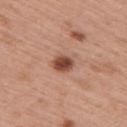| field | value |
|---|---|
| notes | catalogued during a skin exam; not biopsied |
| anatomic site | the upper back |
| lighting | white-light |
| image-analysis metrics | a lesion area of about 4.5 mm² and a shape-asymmetry score of about 0.2 (0 = symmetric); a nevus-likeness score of about 100/100 |
| subject | male, aged approximately 60 |
| image | total-body-photography crop, ~15 mm field of view |
| diameter | ≈2.5 mm |